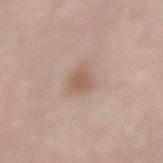The lesion was photographed on a routine skin check and not biopsied; there is no pathology result. The recorded lesion diameter is about 2.5 mm. Imaged with white-light lighting. On the left upper arm. A male patient, aged around 80. A region of skin cropped from a whole-body photographic capture, roughly 15 mm wide. Automated image analysis of the tile measured an area of roughly 4.5 mm², an eccentricity of roughly 0.6, and a symmetry-axis asymmetry near 0.25. It also reported about 9 CIELAB-L* units darker than the surrounding skin. And it measured a border-irregularity rating of about 2/10, a within-lesion color-variation index near 1.5/10, and a peripheral color-asymmetry measure near 0.5.